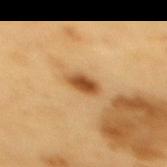Notes:
- biopsy status: no biopsy performed (imaged during a skin exam)
- lighting: cross-polarized
- subject: male, roughly 60 years of age
- imaging modality: ~15 mm tile from a whole-body skin photo
- location: the mid back
- lesion diameter: ~3 mm (longest diameter)